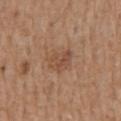Acquisition and patient details:
This image is a 15 mm lesion crop taken from a total-body photograph. The lesion is on the back. This is a white-light tile. Measured at roughly 3.5 mm in maximum diameter. A male subject, approximately 70 years of age.